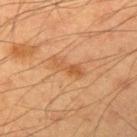Recorded during total-body skin imaging; not selected for excision or biopsy. From the right upper arm. A male patient, aged approximately 55. This image is a 15 mm lesion crop taken from a total-body photograph.